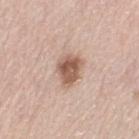follow-up=imaged on a skin check; not biopsied.